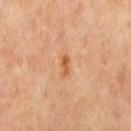Q: Was this lesion biopsied?
A: catalogued during a skin exam; not biopsied
Q: Who is the patient?
A: female, roughly 65 years of age
Q: Where on the body is the lesion?
A: the left thigh
Q: How was this image acquired?
A: ~15 mm tile from a whole-body skin photo